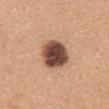  biopsy_status: not biopsied; imaged during a skin examination
  automated_metrics:
    border_irregularity_0_10: 1.0
    color_variation_0_10: 6.0
  lesion_size:
    long_diameter_mm_approx: 4.0
  image:
    source: total-body photography crop
    field_of_view_mm: 15
  site: mid back
  lighting: white-light
  patient:
    sex: female
    age_approx: 30